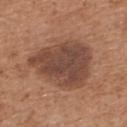  biopsy_status: not biopsied; imaged during a skin examination
  automated_metrics:
    color_variation_0_10: 4.0
    peripheral_color_asymmetry: 1.0
    nevus_likeness_0_100: 50
  image:
    source: total-body photography crop
    field_of_view_mm: 15
  lesion_size:
    long_diameter_mm_approx: 7.5
  lighting: white-light
  site: upper back
  patient:
    sex: male
    age_approx: 70